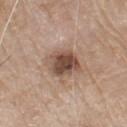<case>
<biopsy_status>not biopsied; imaged during a skin examination</biopsy_status>
<site>chest</site>
<patient>
  <sex>male</sex>
  <age_approx>80</age_approx>
</patient>
<image>
  <source>total-body photography crop</source>
  <field_of_view_mm>15</field_of_view_mm>
</image>
<lighting>white-light</lighting>
<automated_metrics>
  <vs_skin_darker_L>14.0</vs_skin_darker_L>
  <vs_skin_contrast_norm>10.0</vs_skin_contrast_norm>
  <border_irregularity_0_10>1.5</border_irregularity_0_10>
  <peripheral_color_asymmetry>2.0</peripheral_color_asymmetry>
  <nevus_likeness_0_100>60</nevus_likeness_0_100>
  <lesion_detection_confidence_0_100>100</lesion_detection_confidence_0_100>
</automated_metrics>
</case>